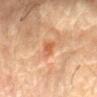<lesion>
  <biopsy_status>not biopsied; imaged during a skin examination</biopsy_status>
  <patient>
    <sex>male</sex>
    <age_approx>85+</age_approx>
  </patient>
  <automated_metrics>
    <cielab_L>52</cielab_L>
    <cielab_a>24</cielab_a>
    <cielab_b>35</cielab_b>
    <vs_skin_darker_L>8.0</vs_skin_darker_L>
    <vs_skin_contrast_norm>6.5</vs_skin_contrast_norm>
    <nevus_likeness_0_100>0</nevus_likeness_0_100>
    <lesion_detection_confidence_0_100>100</lesion_detection_confidence_0_100>
  </automated_metrics>
  <lesion_size>
    <long_diameter_mm_approx>2.5</long_diameter_mm_approx>
  </lesion_size>
  <site>left forearm</site>
  <image>
    <source>total-body photography crop</source>
    <field_of_view_mm>15</field_of_view_mm>
  </image>
</lesion>